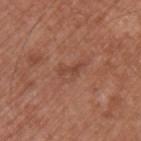Q: What is the anatomic site?
A: the left upper arm
Q: What is the imaging modality?
A: ~15 mm tile from a whole-body skin photo
Q: Patient demographics?
A: male, aged around 65